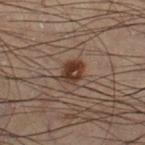Part of a total-body skin-imaging series; this lesion was reviewed on a skin check and was not flagged for biopsy. Cropped from a whole-body photographic skin survey; the tile spans about 15 mm. A male patient, aged around 55. The tile uses cross-polarized illumination. Located on the right lower leg. Automated tile analysis of the lesion measured a footprint of about 5.5 mm², an outline eccentricity of about 0.55 (0 = round, 1 = elongated), and a shape-asymmetry score of about 0.2 (0 = symmetric). And it measured an average lesion color of about L≈28 a*≈15 b*≈21 (CIELAB) and a lesion-to-skin contrast of about 10 (normalized; higher = more distinct). The software also gave a classifier nevus-likeness of about 95/100.